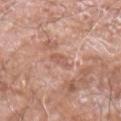  biopsy_status: not biopsied; imaged during a skin examination
  patient:
    sex: male
    age_approx: 60
  site: arm
  image:
    source: total-body photography crop
    field_of_view_mm: 15